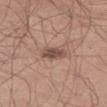Q: What is the imaging modality?
A: 15 mm crop, total-body photography
Q: How was the tile lit?
A: white-light illumination
Q: Lesion size?
A: ≈3.5 mm
Q: Patient demographics?
A: male, aged around 40
Q: What is the anatomic site?
A: the left thigh